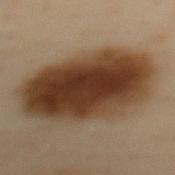Q: Is there a histopathology result?
A: no biopsy performed (imaged during a skin exam)